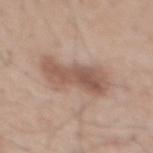Part of a total-body skin-imaging series; this lesion was reviewed on a skin check and was not flagged for biopsy. A male patient in their mid-50s. A lesion tile, about 15 mm wide, cut from a 3D total-body photograph. The lesion-visualizer software estimated a footprint of about 16 mm², an eccentricity of roughly 0.9, and a symmetry-axis asymmetry near 0.25. And it measured a lesion–skin lightness drop of about 11. The software also gave a nevus-likeness score of about 15/100. Captured under white-light illumination. The lesion is on the mid back. Measured at roughly 6.5 mm in maximum diameter.